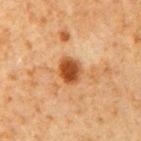Findings:
– biopsy status: total-body-photography surveillance lesion; no biopsy
– lesion diameter: ~3 mm (longest diameter)
– automated metrics: border irregularity of about 1.5 on a 0–10 scale, internal color variation of about 3.5 on a 0–10 scale, and peripheral color asymmetry of about 1; a classifier nevus-likeness of about 100/100 and a detector confidence of about 100 out of 100 that the crop contains a lesion
– anatomic site: the right upper arm
– image source: total-body-photography crop, ~15 mm field of view
– subject: male, roughly 60 years of age
– tile lighting: cross-polarized